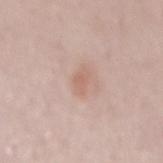{
  "biopsy_status": "not biopsied; imaged during a skin examination",
  "patient": {
    "sex": "female",
    "age_approx": 50
  },
  "lesion_size": {
    "long_diameter_mm_approx": 2.5
  },
  "lighting": "white-light",
  "site": "back",
  "image": {
    "source": "total-body photography crop",
    "field_of_view_mm": 15
  }
}